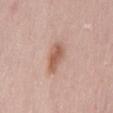Clinical impression:
Recorded during total-body skin imaging; not selected for excision or biopsy.
Context:
An algorithmic analysis of the crop reported a nevus-likeness score of about 60/100. A female subject aged 28–32. This image is a 15 mm lesion crop taken from a total-body photograph. On the mid back. Imaged with white-light lighting. The lesion's longest dimension is about 4 mm.